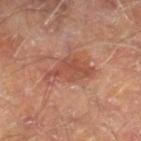workup=total-body-photography surveillance lesion; no biopsy | illumination=cross-polarized | body site=the right lower leg | diameter=~5.5 mm (longest diameter) | patient=male, aged 68 to 72 | imaging modality=total-body-photography crop, ~15 mm field of view.